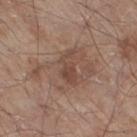Approximately 4 mm at its widest.
A male patient, in their 80s.
The total-body-photography lesion software estimated a footprint of about 5.5 mm², an eccentricity of roughly 0.9, and a symmetry-axis asymmetry near 0.45. And it measured a classifier nevus-likeness of about 0/100 and a detector confidence of about 100 out of 100 that the crop contains a lesion.
The lesion is located on the right thigh.
A lesion tile, about 15 mm wide, cut from a 3D total-body photograph.
Captured under white-light illumination.